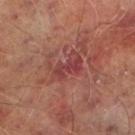– notes — total-body-photography surveillance lesion; no biopsy
– image — ~15 mm tile from a whole-body skin photo
– patient — male, roughly 65 years of age
– site — the left lower leg